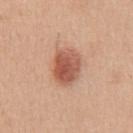Clinical impression: This lesion was catalogued during total-body skin photography and was not selected for biopsy. Clinical summary: The lesion's longest dimension is about 4.5 mm. Located on the abdomen. This is a white-light tile. The patient is a male in their 40s. This image is a 15 mm lesion crop taken from a total-body photograph.